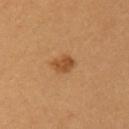Case summary:
* follow-up — imaged on a skin check; not biopsied
* subject — male, aged 18–22
* location — the back
* size — ≈2.5 mm
* image source — total-body-photography crop, ~15 mm field of view
* illumination — cross-polarized illumination